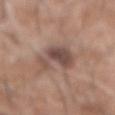biopsy_status: not biopsied; imaged during a skin examination
lesion_size:
  long_diameter_mm_approx: 5.5
lighting: white-light
image:
  source: total-body photography crop
  field_of_view_mm: 15
site: mid back
patient:
  sex: male
  age_approx: 60
automated_metrics:
  area_mm2_approx: 12.0
  shape_asymmetry: 0.4
  nevus_likeness_0_100: 20